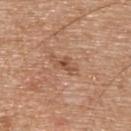{"biopsy_status": "not biopsied; imaged during a skin examination", "patient": {"sex": "male", "age_approx": 65}, "lighting": "white-light", "automated_metrics": {"area_mm2_approx": 3.5, "eccentricity": 0.8, "shape_asymmetry": 0.45, "cielab_L": 52, "cielab_a": 22, "cielab_b": 32, "vs_skin_contrast_norm": 6.5, "border_irregularity_0_10": 4.5, "color_variation_0_10": 3.0, "peripheral_color_asymmetry": 1.5, "nevus_likeness_0_100": 15, "lesion_detection_confidence_0_100": 100}, "site": "upper back", "image": {"source": "total-body photography crop", "field_of_view_mm": 15}, "lesion_size": {"long_diameter_mm_approx": 3.0}}